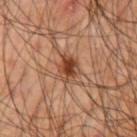This lesion was catalogued during total-body skin photography and was not selected for biopsy.